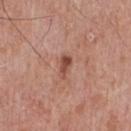Case summary:
* lesion size · about 2.5 mm
* imaging modality · ~15 mm crop, total-body skin-cancer survey
* patient · male, aged 68 to 72
* automated lesion analysis · a mean CIELAB color near L≈48 a*≈24 b*≈29 and a normalized border contrast of about 8.5; border irregularity of about 3.5 on a 0–10 scale, internal color variation of about 1.5 on a 0–10 scale, and radial color variation of about 0.5
* body site · the chest